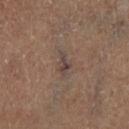{
  "biopsy_status": "not biopsied; imaged during a skin examination",
  "site": "right lower leg",
  "lighting": "cross-polarized",
  "patient": {
    "sex": "male",
    "age_approx": 65
  },
  "image": {
    "source": "total-body photography crop",
    "field_of_view_mm": 15
  }
}